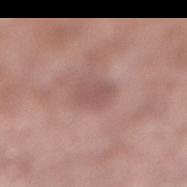Assessment: Imaged during a routine full-body skin examination; the lesion was not biopsied and no histopathology is available. Clinical summary: Longest diameter approximately 3 mm. Located on the leg. A male subject roughly 55 years of age. A 15 mm crop from a total-body photograph taken for skin-cancer surveillance. The lesion-visualizer software estimated a classifier nevus-likeness of about 0/100 and a detector confidence of about 100 out of 100 that the crop contains a lesion. Captured under white-light illumination.